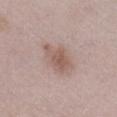{"biopsy_status": "not biopsied; imaged during a skin examination", "image": {"source": "total-body photography crop", "field_of_view_mm": 15}, "automated_metrics": {"area_mm2_approx": 9.5, "eccentricity": 0.75, "shape_asymmetry": 0.2, "cielab_L": 57, "cielab_a": 17, "cielab_b": 23, "vs_skin_darker_L": 9.0, "border_irregularity_0_10": 2.5, "color_variation_0_10": 3.0, "peripheral_color_asymmetry": 1.0, "nevus_likeness_0_100": 55, "lesion_detection_confidence_0_100": 100}, "lesion_size": {"long_diameter_mm_approx": 4.0}, "patient": {"sex": "female", "age_approx": 60}, "site": "right lower leg", "lighting": "white-light"}